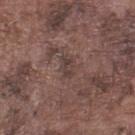Recorded during total-body skin imaging; not selected for excision or biopsy.